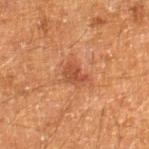notes=no biopsy performed (imaged during a skin exam) | automated metrics=border irregularity of about 3.5 on a 0–10 scale, a color-variation rating of about 2/10, and peripheral color asymmetry of about 0.5; an automated nevus-likeness rating near 10 out of 100 and a detector confidence of about 100 out of 100 that the crop contains a lesion | imaging modality=~15 mm crop, total-body skin-cancer survey | site=the right lower leg | size=about 3 mm | patient=male, roughly 60 years of age.